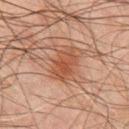Imaged during a routine full-body skin examination; the lesion was not biopsied and no histopathology is available. About 4.5 mm across. From the chest. A 15 mm close-up extracted from a 3D total-body photography capture. Captured under cross-polarized illumination. Automated image analysis of the tile measured a mean CIELAB color near L≈42 a*≈21 b*≈28, a lesion–skin lightness drop of about 8, and a normalized border contrast of about 7. The software also gave a border-irregularity index near 3/10, a color-variation rating of about 2.5/10, and a peripheral color-asymmetry measure near 1. And it measured a lesion-detection confidence of about 100/100. The subject is a male roughly 45 years of age.